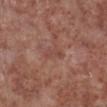{"lighting": "white-light", "patient": {"sex": "male", "age_approx": 55}, "site": "leg", "image": {"source": "total-body photography crop", "field_of_view_mm": 15}, "lesion_size": {"long_diameter_mm_approx": 3.0}}